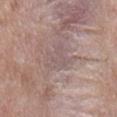Captured under white-light illumination. A male patient aged around 50. The lesion's longest dimension is about 3.5 mm. The lesion is located on the mid back. Cropped from a whole-body photographic skin survey; the tile spans about 15 mm.